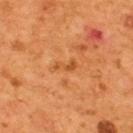Assessment: Imaged during a routine full-body skin examination; the lesion was not biopsied and no histopathology is available. Background: The tile uses cross-polarized illumination. The patient is a male aged around 55. The lesion is located on the upper back. An algorithmic analysis of the crop reported a lesion area of about 2.5 mm², a shape eccentricity near 0.9, and two-axis asymmetry of about 0.45. It also reported a mean CIELAB color near L≈49 a*≈28 b*≈42, a lesion–skin lightness drop of about 8, and a lesion-to-skin contrast of about 6 (normalized; higher = more distinct). The analysis additionally found border irregularity of about 5.5 on a 0–10 scale, a color-variation rating of about 0/10, and peripheral color asymmetry of about 0. A 15 mm close-up extracted from a 3D total-body photography capture. The recorded lesion diameter is about 3 mm.